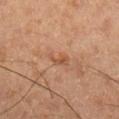No biopsy was performed on this lesion — it was imaged during a full skin examination and was not determined to be concerning. The recorded lesion diameter is about 3 mm. The lesion is located on the leg. A male patient aged 58 to 62. The tile uses cross-polarized illumination. Cropped from a whole-body photographic skin survey; the tile spans about 15 mm.